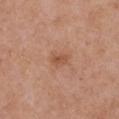The lesion was tiled from a total-body skin photograph and was not biopsied.
Captured under white-light illumination.
From the chest.
A female patient about 40 years old.
A lesion tile, about 15 mm wide, cut from a 3D total-body photograph.
Longest diameter approximately 2.5 mm.